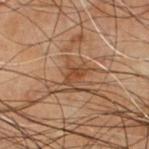• workup: no biopsy performed (imaged during a skin exam)
• imaging modality: total-body-photography crop, ~15 mm field of view
• patient: male, roughly 70 years of age
• tile lighting: cross-polarized illumination
• location: the chest